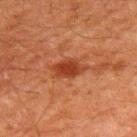Recorded during total-body skin imaging; not selected for excision or biopsy. The lesion-visualizer software estimated an average lesion color of about L≈33 a*≈25 b*≈31 (CIELAB). It also reported a border-irregularity index near 3.5/10, a within-lesion color-variation index near 2.5/10, and a peripheral color-asymmetry measure near 1. A 15 mm close-up tile from a total-body photography series done for melanoma screening. A male subject aged approximately 60. On the upper back. This is a cross-polarized tile. The recorded lesion diameter is about 4.5 mm.